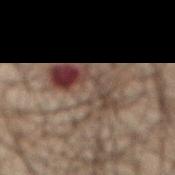Findings:
• biopsy status: total-body-photography surveillance lesion; no biopsy
• automated metrics: a footprint of about 18 mm² and a shape eccentricity near 0.8; an automated nevus-likeness rating near 10 out of 100 and a detector confidence of about 95 out of 100 that the crop contains a lesion
• subject: male, in their mid- to late 60s
• illumination: cross-polarized illumination
• body site: the front of the torso
• lesion size: ≈7 mm
• imaging modality: ~15 mm crop, total-body skin-cancer survey